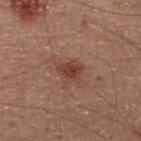Clinical impression:
The lesion was photographed on a routine skin check and not biopsied; there is no pathology result.
Clinical summary:
A male patient about 50 years old. This is a cross-polarized tile. Measured at roughly 3 mm in maximum diameter. The lesion is on the right lower leg. An algorithmic analysis of the crop reported a lesion color around L≈35 a*≈20 b*≈24 in CIELAB and a normalized lesion–skin contrast near 7.5. Cropped from a total-body skin-imaging series; the visible field is about 15 mm.